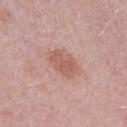Q: Was this lesion biopsied?
A: total-body-photography surveillance lesion; no biopsy
Q: Who is the patient?
A: female, approximately 30 years of age
Q: What kind of image is this?
A: ~15 mm tile from a whole-body skin photo
Q: What is the anatomic site?
A: the right upper arm